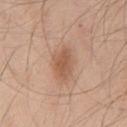Recorded during total-body skin imaging; not selected for excision or biopsy. A male subject aged 43 to 47. Longest diameter approximately 4 mm. A close-up tile cropped from a whole-body skin photograph, about 15 mm across. The lesion-visualizer software estimated an eccentricity of roughly 0.75 and two-axis asymmetry of about 0.2. It also reported a color-variation rating of about 3/10 and peripheral color asymmetry of about 1. And it measured a detector confidence of about 100 out of 100 that the crop contains a lesion. From the chest.